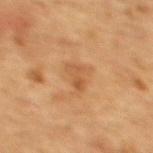* workup · imaged on a skin check; not biopsied